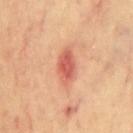workup: imaged on a skin check; not biopsied | subject: male, roughly 70 years of age | size: ~4 mm (longest diameter) | site: the chest | automated metrics: an area of roughly 7 mm², an outline eccentricity of about 0.85 (0 = round, 1 = elongated), and two-axis asymmetry of about 0.3; lesion-presence confidence of about 100/100 | illumination: cross-polarized | image source: 15 mm crop, total-body photography.